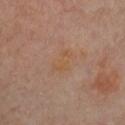notes: catalogued during a skin exam; not biopsied | patient: female, roughly 60 years of age | size: about 3.5 mm | anatomic site: the chest | image: ~15 mm tile from a whole-body skin photo | automated lesion analysis: a lesion area of about 5 mm², an outline eccentricity of about 0.85 (0 = round, 1 = elongated), and a shape-asymmetry score of about 0.45 (0 = symmetric); a border-irregularity index near 5/10; a classifier nevus-likeness of about 0/100 | lighting: cross-polarized illumination.